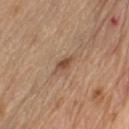biopsy_status: not biopsied; imaged during a skin examination
automated_metrics:
  area_mm2_approx: 2.5
  shape_asymmetry: 0.3
  cielab_L: 49
  cielab_a: 19
  cielab_b: 31
  nevus_likeness_0_100: 65
  lesion_detection_confidence_0_100: 100
lighting: white-light
site: right upper arm
patient:
  sex: male
  age_approx: 70
image:
  source: total-body photography crop
  field_of_view_mm: 15
lesion_size:
  long_diameter_mm_approx: 2.5